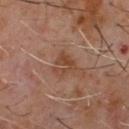The lesion was photographed on a routine skin check and not biopsied; there is no pathology result. Approximately 3 mm at its widest. Imaged with cross-polarized lighting. This image is a 15 mm lesion crop taken from a total-body photograph. A male patient, in their 60s. The lesion is on the chest.